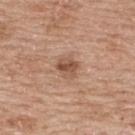This lesion was catalogued during total-body skin photography and was not selected for biopsy. The lesion-visualizer software estimated a lesion area of about 4.5 mm², a shape eccentricity near 0.5, and a symmetry-axis asymmetry near 0.25. And it measured a nevus-likeness score of about 70/100 and lesion-presence confidence of about 100/100. Imaged with white-light lighting. From the upper back. A lesion tile, about 15 mm wide, cut from a 3D total-body photograph. A female patient, aged approximately 60. Longest diameter approximately 2.5 mm.